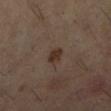Case summary:
- notes · total-body-photography surveillance lesion; no biopsy
- tile lighting · cross-polarized illumination
- body site · the right lower leg
- acquisition · ~15 mm crop, total-body skin-cancer survey
- subject · female, aged approximately 60
- diameter · about 2 mm
- image-analysis metrics · a lesion-detection confidence of about 100/100